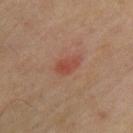<case>
<biopsy_status>not biopsied; imaged during a skin examination</biopsy_status>
<site>upper back</site>
<image>
  <source>total-body photography crop</source>
  <field_of_view_mm>15</field_of_view_mm>
</image>
<lighting>cross-polarized</lighting>
<lesion_size>
  <long_diameter_mm_approx>3.0</long_diameter_mm_approx>
</lesion_size>
<patient>
  <sex>male</sex>
  <age_approx>60</age_approx>
</patient>
</case>